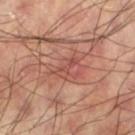Background:
Cropped from a whole-body photographic skin survey; the tile spans about 15 mm. Located on the left thigh. The subject is a male aged 58–62. This is a cross-polarized tile. Measured at roughly 3 mm in maximum diameter. The total-body-photography lesion software estimated a lesion color around L≈49 a*≈26 b*≈27 in CIELAB and about 7 CIELAB-L* units darker than the surrounding skin. The software also gave internal color variation of about 1 on a 0–10 scale and a peripheral color-asymmetry measure near 0.5.